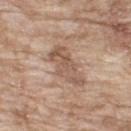Findings:
• notes: imaged on a skin check; not biopsied
• size: ≈5.5 mm
• lighting: white-light illumination
• image: total-body-photography crop, ~15 mm field of view
• patient: male, aged around 65
• location: the upper back